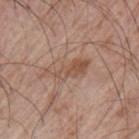follow-up: catalogued during a skin exam; not biopsied | lighting: white-light illumination | size: ≈5.5 mm | site: the left upper arm | patient: male, aged approximately 65 | acquisition: ~15 mm crop, total-body skin-cancer survey.